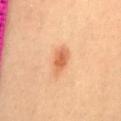Q: Was a biopsy performed?
A: no biopsy performed (imaged during a skin exam)
Q: Lesion location?
A: the lower back
Q: How large is the lesion?
A: about 3.5 mm
Q: What is the imaging modality?
A: 15 mm crop, total-body photography
Q: What are the patient's age and sex?
A: male, about 40 years old
Q: Automated lesion metrics?
A: an area of roughly 5 mm² and a shape eccentricity near 0.85; a lesion color around L≈65 a*≈28 b*≈41 in CIELAB and about 12 CIELAB-L* units darker than the surrounding skin; a nevus-likeness score of about 95/100
Q: How was the tile lit?
A: cross-polarized illumination